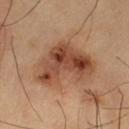Clinical impression: The lesion was photographed on a routine skin check and not biopsied; there is no pathology result. Context: The lesion is on the leg. A region of skin cropped from a whole-body photographic capture, roughly 15 mm wide. The patient is a male aged 58–62. Imaged with cross-polarized lighting. Longest diameter approximately 7.5 mm.